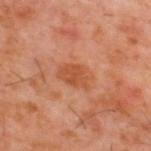<case>
  <biopsy_status>not biopsied; imaged during a skin examination</biopsy_status>
  <patient>
    <sex>male</sex>
    <age_approx>60</age_approx>
  </patient>
  <site>upper back</site>
  <image>
    <source>total-body photography crop</source>
    <field_of_view_mm>15</field_of_view_mm>
  </image>
</case>